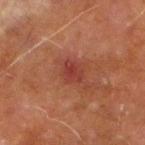Impression: Recorded during total-body skin imaging; not selected for excision or biopsy. Clinical summary: From the right lower leg. A 15 mm crop from a total-body photograph taken for skin-cancer surveillance. The patient is a male approximately 70 years of age.